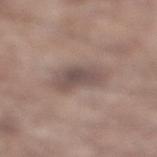Captured during whole-body skin photography for melanoma surveillance; the lesion was not biopsied. This is a white-light tile. Cropped from a total-body skin-imaging series; the visible field is about 15 mm. From the leg. A male patient, aged approximately 55. The recorded lesion diameter is about 4.5 mm.